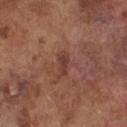Case summary:
– follow-up: no biopsy performed (imaged during a skin exam)
– lighting: white-light
– acquisition: ~15 mm crop, total-body skin-cancer survey
– site: the chest
– automated lesion analysis: a border-irregularity rating of about 3.5/10, a within-lesion color-variation index near 0/10, and a peripheral color-asymmetry measure near 0
– patient: male, in their mid- to late 70s